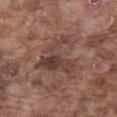Clinical impression:
Part of a total-body skin-imaging series; this lesion was reviewed on a skin check and was not flagged for biopsy.
Image and clinical context:
The subject is a male aged 73 to 77. The lesion is located on the left thigh. An algorithmic analysis of the crop reported a lesion area of about 17 mm², an outline eccentricity of about 0.35 (0 = round, 1 = elongated), and two-axis asymmetry of about 0.65. And it measured a mean CIELAB color near L≈41 a*≈17 b*≈21 and a normalized lesion–skin contrast near 7.5. It also reported an automated nevus-likeness rating near 0 out of 100 and a detector confidence of about 55 out of 100 that the crop contains a lesion. This is a white-light tile. A lesion tile, about 15 mm wide, cut from a 3D total-body photograph.